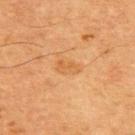The lesion was tiled from a total-body skin photograph and was not biopsied. A male subject about 65 years old. The lesion's longest dimension is about 3 mm. The lesion is on the upper back. Imaged with cross-polarized lighting. A 15 mm close-up tile from a total-body photography series done for melanoma screening.